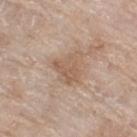follow-up: imaged on a skin check; not biopsied
subject: female, aged 73–77
location: the right thigh
lighting: white-light illumination
size: about 4 mm
acquisition: total-body-photography crop, ~15 mm field of view
image-analysis metrics: a lesion area of about 9 mm², a shape eccentricity near 0.45, and two-axis asymmetry of about 0.35; an average lesion color of about L≈58 a*≈16 b*≈29 (CIELAB), roughly 8 lightness units darker than nearby skin, and a lesion-to-skin contrast of about 6 (normalized; higher = more distinct); a classifier nevus-likeness of about 5/100 and a detector confidence of about 100 out of 100 that the crop contains a lesion